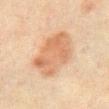Imaged during a routine full-body skin examination; the lesion was not biopsied and no histopathology is available.
From the front of the torso.
The total-body-photography lesion software estimated a lesion area of about 22 mm², an outline eccentricity of about 0.75 (0 = round, 1 = elongated), and a symmetry-axis asymmetry near 0.2. The analysis additionally found a lesion color around L≈54 a*≈18 b*≈31 in CIELAB, roughly 9 lightness units darker than nearby skin, and a lesion-to-skin contrast of about 7 (normalized; higher = more distinct). The software also gave a border-irregularity index near 2.5/10 and a color-variation rating of about 4/10.
Measured at roughly 6.5 mm in maximum diameter.
A roughly 15 mm field-of-view crop from a total-body skin photograph.
The subject is a male about 70 years old.
This is a cross-polarized tile.